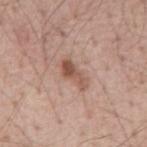{
  "biopsy_status": "not biopsied; imaged during a skin examination",
  "site": "mid back",
  "lesion_size": {
    "long_diameter_mm_approx": 4.0
  },
  "patient": {
    "sex": "male",
    "age_approx": 55
  },
  "image": {
    "source": "total-body photography crop",
    "field_of_view_mm": 15
  }
}